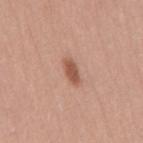Assessment: Part of a total-body skin-imaging series; this lesion was reviewed on a skin check and was not flagged for biopsy. Clinical summary: The subject is a male aged 43–47. A 15 mm close-up extracted from a 3D total-body photography capture. The lesion is located on the back. About 3 mm across. Imaged with white-light lighting.